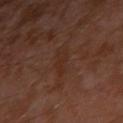The lesion was photographed on a routine skin check and not biopsied; there is no pathology result.
A male subject aged 28–32.
On the arm.
A lesion tile, about 15 mm wide, cut from a 3D total-body photograph.
The tile uses cross-polarized illumination.
Automated tile analysis of the lesion measured about 4 CIELAB-L* units darker than the surrounding skin and a lesion-to-skin contrast of about 5 (normalized; higher = more distinct). And it measured a border-irregularity index near 4/10, a within-lesion color-variation index near 0.5/10, and peripheral color asymmetry of about 0.